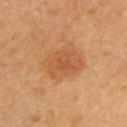Q: Was a biopsy performed?
A: catalogued during a skin exam; not biopsied
Q: What is the anatomic site?
A: the left upper arm
Q: What is the lesion's diameter?
A: about 5 mm
Q: Who is the patient?
A: male, about 50 years old
Q: What is the imaging modality?
A: ~15 mm crop, total-body skin-cancer survey